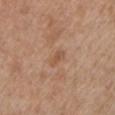Recorded during total-body skin imaging; not selected for excision or biopsy. A male patient in their mid- to late 60s. Imaged with white-light lighting. The recorded lesion diameter is about 2.5 mm. A region of skin cropped from a whole-body photographic capture, roughly 15 mm wide. The lesion is on the left upper arm.